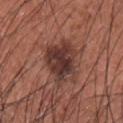Q: Is there a histopathology result?
A: catalogued during a skin exam; not biopsied
Q: How large is the lesion?
A: ≈5 mm
Q: What did automated image analysis measure?
A: a lesion area of about 15 mm², a shape eccentricity near 0.35, and a symmetry-axis asymmetry near 0.3; a lesion–skin lightness drop of about 12
Q: What lighting was used for the tile?
A: white-light
Q: What is the imaging modality?
A: 15 mm crop, total-body photography
Q: Patient demographics?
A: male, about 35 years old
Q: Lesion location?
A: the front of the torso